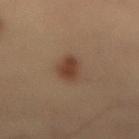Q: Is there a histopathology result?
A: total-body-photography surveillance lesion; no biopsy
Q: Automated lesion metrics?
A: an average lesion color of about L≈32 a*≈15 b*≈24 (CIELAB) and about 8 CIELAB-L* units darker than the surrounding skin; peripheral color asymmetry of about 0.5; an automated nevus-likeness rating near 100 out of 100 and a detector confidence of about 100 out of 100 that the crop contains a lesion
Q: How was the tile lit?
A: cross-polarized illumination
Q: What is the imaging modality?
A: 15 mm crop, total-body photography
Q: What is the anatomic site?
A: the lower back
Q: Lesion size?
A: ≈4 mm
Q: Who is the patient?
A: male, aged 53–57